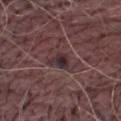  lighting: white-light
  automated_metrics:
    eccentricity: 0.65
    shape_asymmetry: 0.3
    cielab_L: 29
    cielab_a: 15
    cielab_b: 12
    vs_skin_darker_L: 9.0
  site: abdomen
  patient:
    sex: male
    age_approx: 60
  image:
    source: total-body photography crop
    field_of_view_mm: 15
  lesion_size:
    long_diameter_mm_approx: 3.0